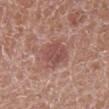<case>
<biopsy_status>not biopsied; imaged during a skin examination</biopsy_status>
<lesion_size>
  <long_diameter_mm_approx>3.5</long_diameter_mm_approx>
</lesion_size>
<site>leg</site>
<image>
  <source>total-body photography crop</source>
  <field_of_view_mm>15</field_of_view_mm>
</image>
<lighting>white-light</lighting>
<patient>
  <sex>male</sex>
  <age_approx>50</age_approx>
</patient>
</case>